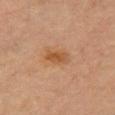| field | value |
|---|---|
| follow-up | no biopsy performed (imaged during a skin exam) |
| anatomic site | the chest |
| illumination | cross-polarized illumination |
| imaging modality | ~15 mm tile from a whole-body skin photo |
| diameter | ≈3 mm |
| subject | female, aged 63 to 67 |